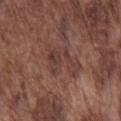Imaged during a routine full-body skin examination; the lesion was not biopsied and no histopathology is available.
A close-up tile cropped from a whole-body skin photograph, about 15 mm across.
This is a white-light tile.
A male patient, roughly 75 years of age.
Measured at roughly 5 mm in maximum diameter.
The lesion is on the chest.